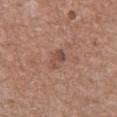Q: Is there a histopathology result?
A: total-body-photography surveillance lesion; no biopsy
Q: What is the lesion's diameter?
A: ≈2.5 mm
Q: What kind of image is this?
A: total-body-photography crop, ~15 mm field of view
Q: Who is the patient?
A: male, aged around 75
Q: Lesion location?
A: the abdomen